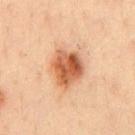No biopsy was performed on this lesion — it was imaged during a full skin examination and was not determined to be concerning. The recorded lesion diameter is about 4.5 mm. Located on the chest. Automated tile analysis of the lesion measured roughly 15 lightness units darker than nearby skin and a normalized lesion–skin contrast near 10. And it measured a classifier nevus-likeness of about 100/100 and a detector confidence of about 100 out of 100 that the crop contains a lesion. A lesion tile, about 15 mm wide, cut from a 3D total-body photograph. A male subject, in their mid-30s.